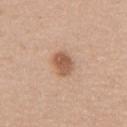The lesion was tiled from a total-body skin photograph and was not biopsied. Approximately 3 mm at its widest. The patient is a female about 30 years old. Captured under white-light illumination. From the chest. A close-up tile cropped from a whole-body skin photograph, about 15 mm across.